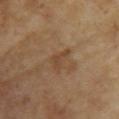– biopsy status — total-body-photography surveillance lesion; no biopsy
– subject — female, aged 73 to 77
– body site — the chest
– image source — 15 mm crop, total-body photography
– lesion diameter — ≈3 mm
– illumination — cross-polarized illumination
– automated metrics — a footprint of about 3 mm², an eccentricity of roughly 0.85, and two-axis asymmetry of about 0.7; an automated nevus-likeness rating near 0 out of 100 and a detector confidence of about 100 out of 100 that the crop contains a lesion